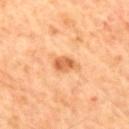The tile uses cross-polarized illumination.
Cropped from a whole-body photographic skin survey; the tile spans about 15 mm.
Approximately 3 mm at its widest.
A male patient aged 58–62.
The lesion is on the mid back.
The lesion-visualizer software estimated a footprint of about 4.5 mm², an eccentricity of roughly 0.8, and a symmetry-axis asymmetry near 0.2. The analysis additionally found a lesion color around L≈63 a*≈27 b*≈43 in CIELAB, a lesion–skin lightness drop of about 12, and a normalized lesion–skin contrast near 8.